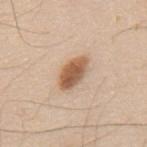<record>
  <biopsy_status>not biopsied; imaged during a skin examination</biopsy_status>
  <site>mid back</site>
  <image>
    <source>total-body photography crop</source>
    <field_of_view_mm>15</field_of_view_mm>
  </image>
  <patient>
    <sex>male</sex>
    <age_approx>60</age_approx>
  </patient>
  <automated_metrics>
    <border_irregularity_0_10>2.0</border_irregularity_0_10>
    <color_variation_0_10>5.0</color_variation_0_10>
    <peripheral_color_asymmetry>1.5</peripheral_color_asymmetry>
  </automated_metrics>
  <lesion_size>
    <long_diameter_mm_approx>4.5</long_diameter_mm_approx>
  </lesion_size>
  <lighting>white-light</lighting>
</record>